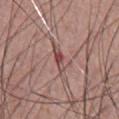Impression: Part of a total-body skin-imaging series; this lesion was reviewed on a skin check and was not flagged for biopsy. Image and clinical context: A 15 mm close-up tile from a total-body photography series done for melanoma screening. A male patient roughly 55 years of age. Located on the chest.